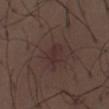follow-up: total-body-photography surveillance lesion; no biopsy
patient: male, aged 28–32
anatomic site: the chest
image: 15 mm crop, total-body photography